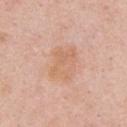Part of a total-body skin-imaging series; this lesion was reviewed on a skin check and was not flagged for biopsy. On the chest. A male patient, about 50 years old. A 15 mm close-up tile from a total-body photography series done for melanoma screening.